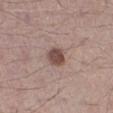notes=no biopsy performed (imaged during a skin exam)
lighting=white-light
subject=male, about 60 years old
body site=the right lower leg
image=total-body-photography crop, ~15 mm field of view